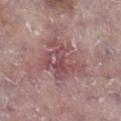location = the right lower leg
lesion diameter = ~6 mm (longest diameter)
tile lighting = white-light illumination
subject = male, aged around 75
image source = ~15 mm crop, total-body skin-cancer survey